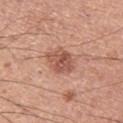Assessment:
Captured during whole-body skin photography for melanoma surveillance; the lesion was not biopsied.
Clinical summary:
The subject is a male aged around 55. About 3.5 mm across. From the left thigh. Automated image analysis of the tile measured an area of roughly 8.5 mm², a shape eccentricity near 0.65, and a symmetry-axis asymmetry near 0.2. It also reported a lesion color around L≈54 a*≈24 b*≈29 in CIELAB, a lesion–skin lightness drop of about 11, and a normalized border contrast of about 7.5. The software also gave border irregularity of about 2 on a 0–10 scale and peripheral color asymmetry of about 1.5. The software also gave a classifier nevus-likeness of about 50/100 and a detector confidence of about 100 out of 100 that the crop contains a lesion. Imaged with white-light lighting. A region of skin cropped from a whole-body photographic capture, roughly 15 mm wide.